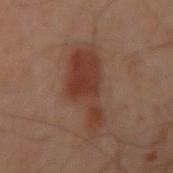A male patient, roughly 50 years of age.
Located on the left arm.
This image is a 15 mm lesion crop taken from a total-body photograph.
The recorded lesion diameter is about 7.5 mm.
An algorithmic analysis of the crop reported a lesion area of about 21 mm², an outline eccentricity of about 0.85 (0 = round, 1 = elongated), and a shape-asymmetry score of about 0.45 (0 = symmetric). The software also gave a border-irregularity index near 5.5/10, a color-variation rating of about 3.5/10, and a peripheral color-asymmetry measure near 1. And it measured a classifier nevus-likeness of about 100/100 and lesion-presence confidence of about 100/100.